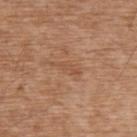Assessment: No biopsy was performed on this lesion — it was imaged during a full skin examination and was not determined to be concerning. Context: The lesion is on the upper back. The tile uses white-light illumination. A male subject, roughly 75 years of age. This image is a 15 mm lesion crop taken from a total-body photograph.